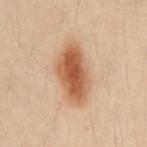workup — catalogued during a skin exam; not biopsied | site — the abdomen | patient — male, aged around 30 | acquisition — ~15 mm tile from a whole-body skin photo | TBP lesion metrics — a shape eccentricity near 0.85; a nevus-likeness score of about 100/100 and a lesion-detection confidence of about 100/100.